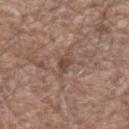Clinical impression: Captured during whole-body skin photography for melanoma surveillance; the lesion was not biopsied. Clinical summary: A lesion tile, about 15 mm wide, cut from a 3D total-body photograph. A male patient aged 68 to 72. The lesion is located on the left forearm.